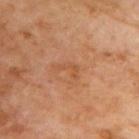workup: total-body-photography surveillance lesion; no biopsy
image source: ~15 mm crop, total-body skin-cancer survey
TBP lesion metrics: an outline eccentricity of about 0.9 (0 = round, 1 = elongated) and two-axis asymmetry of about 0.75; a border-irregularity index near 7.5/10, internal color variation of about 0 on a 0–10 scale, and peripheral color asymmetry of about 0
subject: male, roughly 70 years of age
tile lighting: cross-polarized illumination
lesion size: ≈3 mm
anatomic site: the upper back